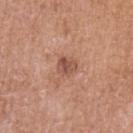biopsy status: no biopsy performed (imaged during a skin exam)
location: the left upper arm
patient: female, approximately 55 years of age
imaging modality: ~15 mm tile from a whole-body skin photo
lighting: white-light illumination
automated metrics: a lesion–skin lightness drop of about 10; border irregularity of about 2 on a 0–10 scale, internal color variation of about 4.5 on a 0–10 scale, and radial color variation of about 1.5; an automated nevus-likeness rating near 30 out of 100 and lesion-presence confidence of about 100/100
lesion diameter: ~2.5 mm (longest diameter)